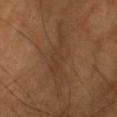acquisition: total-body-photography crop, ~15 mm field of view
anatomic site: the left forearm
patient: male, aged 68 to 72
lesion diameter: about 5.5 mm
image-analysis metrics: a footprint of about 8.5 mm², a shape eccentricity near 0.95, and two-axis asymmetry of about 0.5; an average lesion color of about L≈35 a*≈18 b*≈28 (CIELAB), a lesion–skin lightness drop of about 5, and a normalized lesion–skin contrast near 5